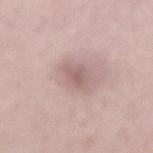Q: Is there a histopathology result?
A: imaged on a skin check; not biopsied
Q: How was the tile lit?
A: white-light illumination
Q: What is the imaging modality?
A: ~15 mm crop, total-body skin-cancer survey
Q: What are the patient's age and sex?
A: male, in their mid- to late 50s
Q: Lesion size?
A: ~3.5 mm (longest diameter)
Q: What is the anatomic site?
A: the lower back